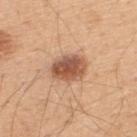notes: total-body-photography surveillance lesion; no biopsy
acquisition: total-body-photography crop, ~15 mm field of view
site: the upper back
patient: male, aged 48–52